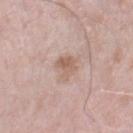Case summary:
- automated lesion analysis: a footprint of about 5.5 mm², an outline eccentricity of about 0.6 (0 = round, 1 = elongated), and a shape-asymmetry score of about 0.3 (0 = symmetric); border irregularity of about 3.5 on a 0–10 scale, internal color variation of about 4 on a 0–10 scale, and radial color variation of about 1.5
- patient: male, in their mid-70s
- tile lighting: white-light
- site: the right thigh
- image: ~15 mm tile from a whole-body skin photo
- diameter: about 3 mm